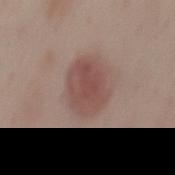{"image": {"source": "total-body photography crop", "field_of_view_mm": 15}, "lighting": "white-light", "site": "mid back", "patient": {"sex": "female", "age_approx": 45}, "lesion_size": {"long_diameter_mm_approx": 5.0}}